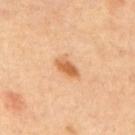workup = imaged on a skin check; not biopsied | site = the mid back | acquisition = total-body-photography crop, ~15 mm field of view | illumination = cross-polarized | lesion size = ≈3.5 mm | subject = male, roughly 70 years of age.